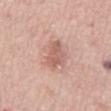Cropped from a total-body skin-imaging series; the visible field is about 15 mm. Located on the front of the torso. Imaged with white-light lighting. Longest diameter approximately 4.5 mm. Automated tile analysis of the lesion measured a mean CIELAB color near L≈60 a*≈23 b*≈26 and about 10 CIELAB-L* units darker than the surrounding skin. The software also gave border irregularity of about 4 on a 0–10 scale and a peripheral color-asymmetry measure near 1. A male patient, aged 53 to 57.